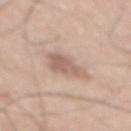Assessment: No biopsy was performed on this lesion — it was imaged during a full skin examination and was not determined to be concerning. Background: A lesion tile, about 15 mm wide, cut from a 3D total-body photograph. The subject is a male in their mid-60s. From the back.